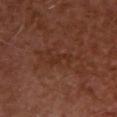Q: Was this lesion biopsied?
A: no biopsy performed (imaged during a skin exam)
Q: Illumination type?
A: cross-polarized
Q: Patient demographics?
A: male, approximately 50 years of age
Q: What did automated image analysis measure?
A: a footprint of about 2.5 mm², a shape eccentricity near 0.95, and two-axis asymmetry of about 0.35; a border-irregularity rating of about 4.5/10, a within-lesion color-variation index near 0/10, and a peripheral color-asymmetry measure near 0
Q: Lesion size?
A: ~3 mm (longest diameter)
Q: What kind of image is this?
A: 15 mm crop, total-body photography
Q: Where on the body is the lesion?
A: the upper back